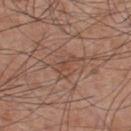Imaged during a routine full-body skin examination; the lesion was not biopsied and no histopathology is available.
The subject is a male in their mid-50s.
On the chest.
A lesion tile, about 15 mm wide, cut from a 3D total-body photograph.